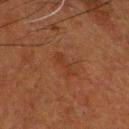A lesion tile, about 15 mm wide, cut from a 3D total-body photograph. The lesion is located on the head or neck. The patient is a male aged 58–62.